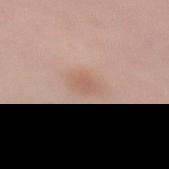This lesion was catalogued during total-body skin photography and was not selected for biopsy. A female patient, aged 18 to 22. Measured at roughly 3 mm in maximum diameter. The lesion is on the right forearm. A 15 mm close-up tile from a total-body photography series done for melanoma screening. Imaged with white-light lighting. An algorithmic analysis of the crop reported an outline eccentricity of about 0.65 (0 = round, 1 = elongated) and two-axis asymmetry of about 0.2. The software also gave border irregularity of about 2 on a 0–10 scale, internal color variation of about 1.5 on a 0–10 scale, and radial color variation of about 0.5.